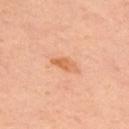Q: Was this lesion biopsied?
A: imaged on a skin check; not biopsied
Q: Where on the body is the lesion?
A: the upper back
Q: What is the imaging modality?
A: ~15 mm tile from a whole-body skin photo
Q: Who is the patient?
A: male, aged 63 to 67